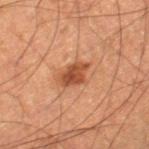| feature | finding |
|---|---|
| notes | imaged on a skin check; not biopsied |
| automated metrics | a footprint of about 5.5 mm², an eccentricity of roughly 0.75, and a shape-asymmetry score of about 0.25 (0 = symmetric); an average lesion color of about L≈45 a*≈26 b*≈34 (CIELAB), about 13 CIELAB-L* units darker than the surrounding skin, and a normalized border contrast of about 9.5; border irregularity of about 2.5 on a 0–10 scale and a peripheral color-asymmetry measure near 1; a classifier nevus-likeness of about 85/100 and a lesion-detection confidence of about 100/100 |
| patient | male, aged 58 to 62 |
| imaging modality | total-body-photography crop, ~15 mm field of view |
| anatomic site | the right thigh |